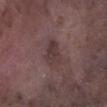Impression:
The lesion was tiled from a total-body skin photograph and was not biopsied.
Acquisition and patient details:
From the left lower leg. Captured under white-light illumination. A lesion tile, about 15 mm wide, cut from a 3D total-body photograph. The recorded lesion diameter is about 3.5 mm. A male subject roughly 75 years of age. Automated image analysis of the tile measured a mean CIELAB color near L≈36 a*≈17 b*≈16, a lesion–skin lightness drop of about 7, and a normalized border contrast of about 6.5. The software also gave a border-irregularity index near 3/10, a within-lesion color-variation index near 3/10, and a peripheral color-asymmetry measure near 1.